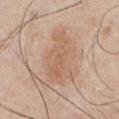automated lesion analysis = an area of roughly 18 mm² and a shape eccentricity near 0.85; an average lesion color of about L≈61 a*≈19 b*≈31 (CIELAB) and a lesion–skin lightness drop of about 7; an automated nevus-likeness rating near 5 out of 100 and a lesion-detection confidence of about 100/100
subject = male, roughly 45 years of age
imaging modality = ~15 mm tile from a whole-body skin photo
lighting = white-light
body site = the chest